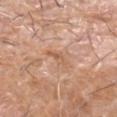No biopsy was performed on this lesion — it was imaged during a full skin examination and was not determined to be concerning.
The lesion's longest dimension is about 3.5 mm.
Automated image analysis of the tile measured a lesion color around L≈60 a*≈21 b*≈33 in CIELAB and about 7 CIELAB-L* units darker than the surrounding skin. The analysis additionally found a within-lesion color-variation index near 1/10 and peripheral color asymmetry of about 0.
Cropped from a whole-body photographic skin survey; the tile spans about 15 mm.
The tile uses white-light illumination.
From the left forearm.
A male patient, aged 73–77.